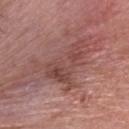| field | value |
|---|---|
| acquisition | 15 mm crop, total-body photography |
| patient | female, about 75 years old |
| site | the head or neck |